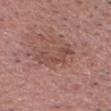No biopsy was performed on this lesion — it was imaged during a full skin examination and was not determined to be concerning. Longest diameter approximately 5 mm. A 15 mm crop from a total-body photograph taken for skin-cancer surveillance. From the head or neck. Imaged with white-light lighting. A male subject, aged 53 to 57.